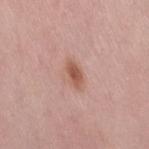Clinical impression: The lesion was tiled from a total-body skin photograph and was not biopsied. Clinical summary: A lesion tile, about 15 mm wide, cut from a 3D total-body photograph. A female subject, aged around 50. Automated tile analysis of the lesion measured a lesion area of about 4.5 mm², an eccentricity of roughly 0.85, and a shape-asymmetry score of about 0.2 (0 = symmetric). The analysis additionally found lesion-presence confidence of about 100/100. Longest diameter approximately 3.5 mm. Captured under white-light illumination. The lesion is located on the right thigh.